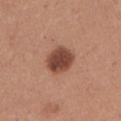The lesion was tiled from a total-body skin photograph and was not biopsied. From the left upper arm. A 15 mm crop from a total-body photograph taken for skin-cancer surveillance. The recorded lesion diameter is about 3.5 mm. The patient is a male aged around 35. The tile uses white-light illumination.